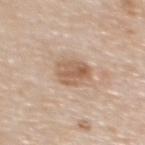Assessment: This lesion was catalogued during total-body skin photography and was not selected for biopsy. Acquisition and patient details: Imaged with white-light lighting. A 15 mm close-up extracted from a 3D total-body photography capture. A female patient aged around 50. Located on the upper back. Automated image analysis of the tile measured a lesion color around L≈60 a*≈18 b*≈31 in CIELAB and about 10 CIELAB-L* units darker than the surrounding skin. It also reported a nevus-likeness score of about 25/100 and lesion-presence confidence of about 100/100.